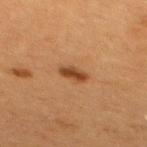{"biopsy_status": "not biopsied; imaged during a skin examination", "automated_metrics": {"border_irregularity_0_10": 2.5, "color_variation_0_10": 1.5, "peripheral_color_asymmetry": 0.5, "nevus_likeness_0_100": 95, "lesion_detection_confidence_0_100": 100}, "site": "upper back", "lesion_size": {"long_diameter_mm_approx": 3.0}, "image": {"source": "total-body photography crop", "field_of_view_mm": 15}, "patient": {"sex": "female", "age_approx": 40}}